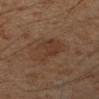biopsy status: imaged on a skin check; not biopsied | body site: the left forearm | patient: male, in their mid- to late 40s | diameter: ≈4.5 mm | imaging modality: ~15 mm crop, total-body skin-cancer survey.